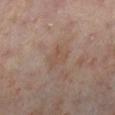Clinical impression: Part of a total-body skin-imaging series; this lesion was reviewed on a skin check and was not flagged for biopsy. Image and clinical context: The patient is a female aged around 50. Cropped from a total-body skin-imaging series; the visible field is about 15 mm. The lesion is located on the right lower leg. An algorithmic analysis of the crop reported a lesion area of about 4.5 mm² and two-axis asymmetry of about 0.4. The analysis additionally found a mean CIELAB color near L≈48 a*≈16 b*≈26, roughly 5 lightness units darker than nearby skin, and a normalized lesion–skin contrast near 5. The software also gave a color-variation rating of about 1.5/10 and peripheral color asymmetry of about 0.5. The software also gave a nevus-likeness score of about 0/100 and lesion-presence confidence of about 100/100.